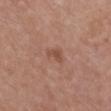Clinical impression: Imaged during a routine full-body skin examination; the lesion was not biopsied and no histopathology is available. Background: A close-up tile cropped from a whole-body skin photograph, about 15 mm across. About 2.5 mm across. A female patient about 65 years old. Located on the chest. The lesion-visualizer software estimated a lesion area of about 3 mm², an outline eccentricity of about 0.85 (0 = round, 1 = elongated), and two-axis asymmetry of about 0.4. It also reported a mean CIELAB color near L≈49 a*≈22 b*≈29, roughly 7 lightness units darker than nearby skin, and a lesion-to-skin contrast of about 6 (normalized; higher = more distinct).